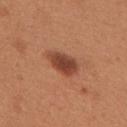This lesion was catalogued during total-body skin photography and was not selected for biopsy.
A female patient, aged around 30.
Imaged with white-light lighting.
Cropped from a whole-body photographic skin survey; the tile spans about 15 mm.
Approximately 4.5 mm at its widest.
Located on the upper back.
An algorithmic analysis of the crop reported a lesion area of about 8 mm², an outline eccentricity of about 0.85 (0 = round, 1 = elongated), and a shape-asymmetry score of about 0.2 (0 = symmetric). And it measured a lesion color around L≈44 a*≈26 b*≈32 in CIELAB, about 13 CIELAB-L* units darker than the surrounding skin, and a normalized lesion–skin contrast near 10. The software also gave a border-irregularity rating of about 2.5/10, a color-variation rating of about 3.5/10, and peripheral color asymmetry of about 1.